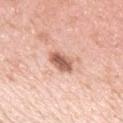biopsy status: no biopsy performed (imaged during a skin exam); body site: the left upper arm; lighting: white-light illumination; subject: female, aged around 40; size: ≈3.5 mm; TBP lesion metrics: a lesion color around L≈60 a*≈24 b*≈29 in CIELAB, about 16 CIELAB-L* units darker than the surrounding skin, and a normalized lesion–skin contrast near 10; imaging modality: total-body-photography crop, ~15 mm field of view.